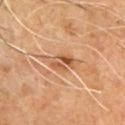<lesion>
  <biopsy_status>not biopsied; imaged during a skin examination</biopsy_status>
  <site>chest</site>
  <image>
    <source>total-body photography crop</source>
    <field_of_view_mm>15</field_of_view_mm>
  </image>
  <lighting>cross-polarized</lighting>
  <patient>
    <sex>male</sex>
    <age_approx>60</age_approx>
  </patient>
</lesion>